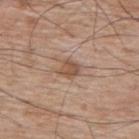A male subject, approximately 70 years of age.
Located on the upper back.
Cropped from a whole-body photographic skin survey; the tile spans about 15 mm.